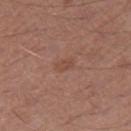{"site": "right thigh", "patient": {"sex": "male", "age_approx": 65}, "image": {"source": "total-body photography crop", "field_of_view_mm": 15}}